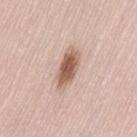patient: female, aged approximately 65
body site: the lower back
size: ~4.5 mm (longest diameter)
image: ~15 mm crop, total-body skin-cancer survey